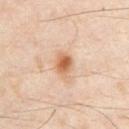biopsy status: catalogued during a skin exam; not biopsied | image: 15 mm crop, total-body photography | site: the chest | patient: male, roughly 35 years of age | lesion size: ~3 mm (longest diameter) | TBP lesion metrics: an average lesion color of about L≈61 a*≈21 b*≈35 (CIELAB), about 12 CIELAB-L* units darker than the surrounding skin, and a normalized border contrast of about 8.5; a classifier nevus-likeness of about 95/100 and a lesion-detection confidence of about 100/100.